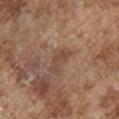Clinical impression:
The lesion was tiled from a total-body skin photograph and was not biopsied.
Acquisition and patient details:
The total-body-photography lesion software estimated a mean CIELAB color near L≈46 a*≈18 b*≈28, about 8 CIELAB-L* units darker than the surrounding skin, and a lesion-to-skin contrast of about 6 (normalized; higher = more distinct). It also reported a border-irregularity rating of about 6/10, a color-variation rating of about 0.5/10, and peripheral color asymmetry of about 0. It also reported an automated nevus-likeness rating near 0 out of 100 and a lesion-detection confidence of about 100/100. Cropped from a whole-body photographic skin survey; the tile spans about 15 mm. The lesion's longest dimension is about 3.5 mm. A male subject aged around 75. From the right upper arm. This is a white-light tile.